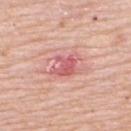Notes:
* workup: no biopsy performed (imaged during a skin exam)
* illumination: white-light
* acquisition: 15 mm crop, total-body photography
* body site: the upper back
* subject: male, roughly 80 years of age
* lesion diameter: ~3.5 mm (longest diameter)
* TBP lesion metrics: a classifier nevus-likeness of about 0/100 and a lesion-detection confidence of about 100/100